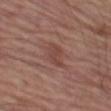This lesion was catalogued during total-body skin photography and was not selected for biopsy. A male subject roughly 65 years of age. From the left thigh. A region of skin cropped from a whole-body photographic capture, roughly 15 mm wide.